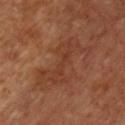| field | value |
|---|---|
| biopsy status | no biopsy performed (imaged during a skin exam) |
| subject | male, approximately 50 years of age |
| image-analysis metrics | an eccentricity of roughly 0.9 and a symmetry-axis asymmetry near 0.55; about 6 CIELAB-L* units darker than the surrounding skin and a normalized border contrast of about 5; a border-irregularity index near 9/10, a within-lesion color-variation index near 2/10, and a peripheral color-asymmetry measure near 0.5 |
| diameter | about 7 mm |
| anatomic site | the chest |
| tile lighting | cross-polarized illumination |
| image source | ~15 mm tile from a whole-body skin photo |